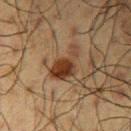The lesion-visualizer software estimated a mean CIELAB color near L≈29 a*≈17 b*≈25, a lesion–skin lightness drop of about 10, and a normalized border contrast of about 10.5. The analysis additionally found an automated nevus-likeness rating near 100 out of 100 and lesion-presence confidence of about 100/100.
The lesion is on the arm.
The tile uses cross-polarized illumination.
A male subject about 60 years old.
Cropped from a total-body skin-imaging series; the visible field is about 15 mm.
The lesion's longest dimension is about 5 mm.